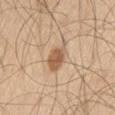<tbp_lesion>
<biopsy_status>not biopsied; imaged during a skin examination</biopsy_status>
<site>left thigh</site>
<lighting>white-light</lighting>
<lesion_size>
  <long_diameter_mm_approx>4.0</long_diameter_mm_approx>
</lesion_size>
<image>
  <source>total-body photography crop</source>
  <field_of_view_mm>15</field_of_view_mm>
</image>
<patient>
  <sex>male</sex>
  <age_approx>60</age_approx>
</patient>
</tbp_lesion>